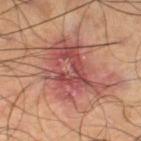Captured under cross-polarized illumination. A 15 mm close-up extracted from a 3D total-body photography capture. The lesion's longest dimension is about 8.5 mm. The subject is a male aged 58–62. The lesion is on the left thigh.